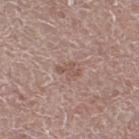<lesion>
<biopsy_status>not biopsied; imaged during a skin examination</biopsy_status>
<automated_metrics>
  <area_mm2_approx>3.0</area_mm2_approx>
  <eccentricity>0.85</eccentricity>
  <shape_asymmetry>0.5</shape_asymmetry>
  <cielab_L>53</cielab_L>
  <cielab_a>18</cielab_a>
  <cielab_b>24</cielab_b>
  <vs_skin_darker_L>7.0</vs_skin_darker_L>
  <vs_skin_contrast_norm>6.0</vs_skin_contrast_norm>
  <lesion_detection_confidence_0_100>100</lesion_detection_confidence_0_100>
</automated_metrics>
<site>right thigh</site>
<image>
  <source>total-body photography crop</source>
  <field_of_view_mm>15</field_of_view_mm>
</image>
<patient>
  <sex>female</sex>
  <age_approx>65</age_approx>
</patient>
<lesion_size>
  <long_diameter_mm_approx>2.5</long_diameter_mm_approx>
</lesion_size>
<lighting>white-light</lighting>
</lesion>